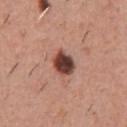Imaged during a routine full-body skin examination; the lesion was not biopsied and no histopathology is available.
Automated tile analysis of the lesion measured a mean CIELAB color near L≈42 a*≈23 b*≈25, about 20 CIELAB-L* units darker than the surrounding skin, and a lesion-to-skin contrast of about 14 (normalized; higher = more distinct).
About 3.5 mm across.
The subject is a male roughly 45 years of age.
The lesion is on the chest.
Captured under white-light illumination.
Cropped from a total-body skin-imaging series; the visible field is about 15 mm.